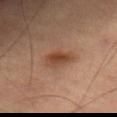workup = no biopsy performed (imaged during a skin exam); tile lighting = cross-polarized illumination; patient = male, about 70 years old; image = total-body-photography crop, ~15 mm field of view; automated lesion analysis = an automated nevus-likeness rating near 90 out of 100 and a detector confidence of about 100 out of 100 that the crop contains a lesion; size = about 4 mm; anatomic site = the right thigh.